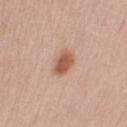follow-up: catalogued during a skin exam; not biopsied | illumination: white-light illumination | lesion diameter: about 3 mm | body site: the right upper arm | patient: male, approximately 60 years of age | automated lesion analysis: a footprint of about 5.5 mm² and an outline eccentricity of about 0.65 (0 = round, 1 = elongated); a mean CIELAB color near L≈56 a*≈23 b*≈30, a lesion–skin lightness drop of about 13, and a normalized border contrast of about 9; a classifier nevus-likeness of about 95/100 and a detector confidence of about 100 out of 100 that the crop contains a lesion | image: 15 mm crop, total-body photography.